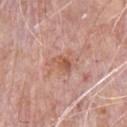Case summary:
– workup — total-body-photography surveillance lesion; no biopsy
– site — the chest
– image source — ~15 mm tile from a whole-body skin photo
– automated lesion analysis — a lesion color around L≈56 a*≈23 b*≈30 in CIELAB, a lesion–skin lightness drop of about 9, and a normalized border contrast of about 6.5; border irregularity of about 3.5 on a 0–10 scale, a within-lesion color-variation index near 4/10, and peripheral color asymmetry of about 1.5; an automated nevus-likeness rating near 5 out of 100 and a detector confidence of about 100 out of 100 that the crop contains a lesion
– lesion size — ~3 mm (longest diameter)
– subject — male, approximately 70 years of age
– tile lighting — white-light